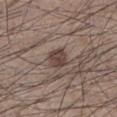subject: male, aged 33 to 37 | size: about 3 mm | automated lesion analysis: border irregularity of about 2.5 on a 0–10 scale, a within-lesion color-variation index near 2.5/10, and radial color variation of about 1; an automated nevus-likeness rating near 75 out of 100 | body site: the left lower leg | tile lighting: white-light illumination | image source: total-body-photography crop, ~15 mm field of view.